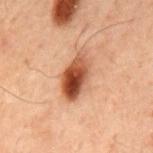TBP lesion metrics=an average lesion color of about L≈40 a*≈21 b*≈28 (CIELAB), about 14 CIELAB-L* units darker than the surrounding skin, and a normalized lesion–skin contrast near 11.5; a border-irregularity index near 2.5/10 and a within-lesion color-variation index near 9.5/10
image=15 mm crop, total-body photography
subject=male, aged 58 to 62
anatomic site=the upper back
illumination=cross-polarized illumination